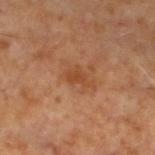biopsy_status: not biopsied; imaged during a skin examination
image:
  source: total-body photography crop
  field_of_view_mm: 15
site: left lower leg
patient:
  sex: male
  age_approx: 60
lighting: cross-polarized
lesion_size:
  long_diameter_mm_approx: 3.0
automated_metrics:
  area_mm2_approx: 3.5
  eccentricity: 0.8
  shape_asymmetry: 0.45
  cielab_L: 43
  cielab_a: 23
  cielab_b: 34
  vs_skin_darker_L: 7.0
  vs_skin_contrast_norm: 6.0
  border_irregularity_0_10: 4.5
  color_variation_0_10: 1.0
  peripheral_color_asymmetry: 0.5